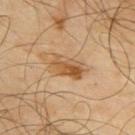Imaged during a routine full-body skin examination; the lesion was not biopsied and no histopathology is available.
The total-body-photography lesion software estimated an area of roughly 7.5 mm², a shape eccentricity near 0.85, and a symmetry-axis asymmetry near 0.2. The software also gave a lesion–skin lightness drop of about 10 and a lesion-to-skin contrast of about 8 (normalized; higher = more distinct). The analysis additionally found a border-irregularity index near 2.5/10 and peripheral color asymmetry of about 2.5. The software also gave a classifier nevus-likeness of about 65/100 and a lesion-detection confidence of about 100/100.
Imaged with cross-polarized lighting.
On the back.
A male subject, approximately 65 years of age.
The lesion's longest dimension is about 4 mm.
A lesion tile, about 15 mm wide, cut from a 3D total-body photograph.